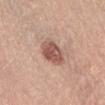notes=imaged on a skin check; not biopsied
location=the abdomen
subject=female, aged approximately 65
diameter=about 3.5 mm
tile lighting=white-light illumination
imaging modality=~15 mm crop, total-body skin-cancer survey